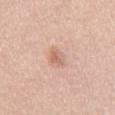Part of a total-body skin-imaging series; this lesion was reviewed on a skin check and was not flagged for biopsy. A 15 mm crop from a total-body photograph taken for skin-cancer surveillance. A female patient about 40 years old. The lesion is located on the chest. Measured at roughly 3 mm in maximum diameter. Captured under white-light illumination.